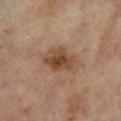Automated tile analysis of the lesion measured an area of roughly 10 mm² and a shape eccentricity near 0.65. It also reported a normalized border contrast of about 8.5. The recorded lesion diameter is about 4 mm. The lesion is located on the right lower leg. A close-up tile cropped from a whole-body skin photograph, about 15 mm across. The subject is a female roughly 75 years of age. Imaged with cross-polarized lighting.